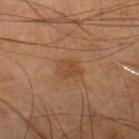  lesion_size:
    long_diameter_mm_approx: 3.0
  lighting: cross-polarized
  image:
    source: total-body photography crop
    field_of_view_mm: 15
  automated_metrics:
    cielab_L: 44
    cielab_a: 21
    cielab_b: 34
    vs_skin_contrast_norm: 5.5
    color_variation_0_10: 2.0
    peripheral_color_asymmetry: 0.5
  site: right lower leg
  patient:
    sex: male
    age_approx: 70